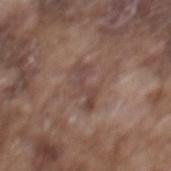This lesion was catalogued during total-body skin photography and was not selected for biopsy. A male subject aged around 75. Longest diameter approximately 4.5 mm. The lesion is located on the mid back. Cropped from a total-body skin-imaging series; the visible field is about 15 mm. The tile uses white-light illumination.